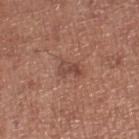Q: Was a biopsy performed?
A: imaged on a skin check; not biopsied
Q: What is the imaging modality?
A: 15 mm crop, total-body photography
Q: What lighting was used for the tile?
A: white-light illumination
Q: Lesion location?
A: the right lower leg
Q: What is the lesion's diameter?
A: ≈3 mm
Q: What are the patient's age and sex?
A: male, roughly 70 years of age
Q: Automated lesion metrics?
A: a lesion area of about 4 mm², an outline eccentricity of about 0.8 (0 = round, 1 = elongated), and a shape-asymmetry score of about 0.35 (0 = symmetric); an average lesion color of about L≈46 a*≈22 b*≈26 (CIELAB), roughly 8 lightness units darker than nearby skin, and a normalized border contrast of about 6.5; a border-irregularity rating of about 4/10, internal color variation of about 3 on a 0–10 scale, and peripheral color asymmetry of about 1; a classifier nevus-likeness of about 0/100